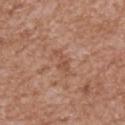Q: Lesion location?
A: the upper back
Q: How large is the lesion?
A: about 3.5 mm
Q: What are the patient's age and sex?
A: male, approximately 65 years of age
Q: How was this image acquired?
A: 15 mm crop, total-body photography